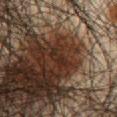notes: total-body-photography surveillance lesion; no biopsy | patient: male, approximately 45 years of age | anatomic site: the abdomen | imaging modality: total-body-photography crop, ~15 mm field of view.